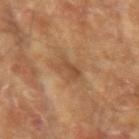notes = no biopsy performed (imaged during a skin exam)
anatomic site = the left upper arm
patient = male, aged 63 to 67
lesion diameter = ≈3 mm
image = total-body-photography crop, ~15 mm field of view